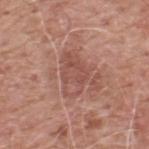follow-up: catalogued during a skin exam; not biopsied | imaging modality: ~15 mm tile from a whole-body skin photo | site: the upper back | patient: male, approximately 60 years of age | illumination: white-light.